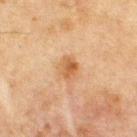This lesion was catalogued during total-body skin photography and was not selected for biopsy.
The lesion is on the chest.
Captured under cross-polarized illumination.
A 15 mm close-up extracted from a 3D total-body photography capture.
A male subject, in their mid-60s.
About 3 mm across.
The total-body-photography lesion software estimated a lesion area of about 5 mm², an outline eccentricity of about 0.7 (0 = round, 1 = elongated), and a symmetry-axis asymmetry near 0.2. The analysis additionally found a nevus-likeness score of about 70/100 and a lesion-detection confidence of about 100/100.